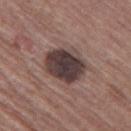Context: This image is a 15 mm lesion crop taken from a total-body photograph. The lesion is located on the left thigh. The recorded lesion diameter is about 5 mm. This is a white-light tile. The subject is a male aged approximately 65. The total-body-photography lesion software estimated a mean CIELAB color near L≈37 a*≈15 b*≈16, roughly 16 lightness units darker than nearby skin, and a normalized lesion–skin contrast near 13. The analysis additionally found border irregularity of about 1.5 on a 0–10 scale, a color-variation rating of about 5/10, and radial color variation of about 1.5. The software also gave a nevus-likeness score of about 0/100 and a detector confidence of about 100 out of 100 that the crop contains a lesion.